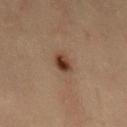diameter=≈2.5 mm
image source=total-body-photography crop, ~15 mm field of view
automated metrics=a lesion color around L≈32 a*≈16 b*≈24 in CIELAB and a normalized lesion–skin contrast near 10; a nevus-likeness score of about 100/100 and lesion-presence confidence of about 100/100
body site=the abdomen
patient=female, approximately 40 years of age
lighting=cross-polarized illumination